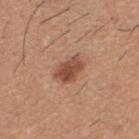Captured during whole-body skin photography for melanoma surveillance; the lesion was not biopsied.
The subject is a male in their 60s.
The recorded lesion diameter is about 3.5 mm.
This is a white-light tile.
A 15 mm close-up extracted from a 3D total-body photography capture.
The lesion is located on the upper back.